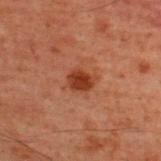Case summary:
* biopsy status · no biopsy performed (imaged during a skin exam)
* size · ≈2.5 mm
* image source · 15 mm crop, total-body photography
* subject · male, about 60 years old
* site · the back
* lighting · cross-polarized